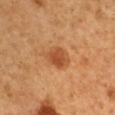Clinical impression:
Imaged during a routine full-body skin examination; the lesion was not biopsied and no histopathology is available.
Acquisition and patient details:
This is a cross-polarized tile. A 15 mm crop from a total-body photograph taken for skin-cancer surveillance. A male subject roughly 50 years of age. On the arm.